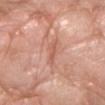Recorded during total-body skin imaging; not selected for excision or biopsy.
The lesion is located on the left forearm.
The lesion's longest dimension is about 3 mm.
Automated image analysis of the tile measured a mean CIELAB color near L≈59 a*≈26 b*≈31, roughly 8 lightness units darker than nearby skin, and a lesion-to-skin contrast of about 5.5 (normalized; higher = more distinct).
A lesion tile, about 15 mm wide, cut from a 3D total-body photograph.
A male subject aged 58–62.